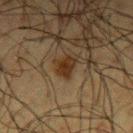This lesion was catalogued during total-body skin photography and was not selected for biopsy.